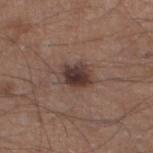{
  "biopsy_status": "not biopsied; imaged during a skin examination",
  "patient": {
    "sex": "male",
    "age_approx": 45
  },
  "site": "left lower leg",
  "automated_metrics": {
    "area_mm2_approx": 8.0,
    "eccentricity": 0.65,
    "shape_asymmetry": 0.2,
    "nevus_likeness_0_100": 85,
    "lesion_detection_confidence_0_100": 100
  },
  "lighting": "white-light",
  "image": {
    "source": "total-body photography crop",
    "field_of_view_mm": 15
  }
}